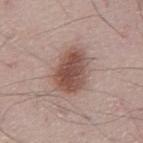The lesion was photographed on a routine skin check and not biopsied; there is no pathology result.
Approximately 5.5 mm at its widest.
On the abdomen.
A male subject, approximately 65 years of age.
Cropped from a whole-body photographic skin survey; the tile spans about 15 mm.
Captured under white-light illumination.
Automated tile analysis of the lesion measured an eccentricity of roughly 0.7. The analysis additionally found about 13 CIELAB-L* units darker than the surrounding skin and a normalized lesion–skin contrast near 9. And it measured lesion-presence confidence of about 100/100.